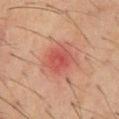A 15 mm crop from a total-body photograph taken for skin-cancer surveillance.
The recorded lesion diameter is about 4 mm.
A male patient aged 58–62.
The lesion is located on the chest.
The tile uses cross-polarized illumination.